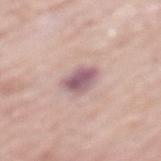An algorithmic analysis of the crop reported an area of roughly 7 mm², a shape eccentricity near 0.65, and two-axis asymmetry of about 0.2. The analysis additionally found border irregularity of about 2 on a 0–10 scale, a color-variation rating of about 4.5/10, and peripheral color asymmetry of about 1.5. The subject is a male approximately 80 years of age. On the mid back. This image is a 15 mm lesion crop taken from a total-body photograph. About 3.5 mm across. The tile uses white-light illumination.